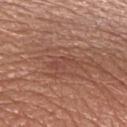Clinical impression:
Part of a total-body skin-imaging series; this lesion was reviewed on a skin check and was not flagged for biopsy.
Clinical summary:
On the left upper arm. Automated image analysis of the tile measured a footprint of about 4 mm², an outline eccentricity of about 0.95 (0 = round, 1 = elongated), and two-axis asymmetry of about 0.55. The software also gave a within-lesion color-variation index near 1/10 and radial color variation of about 0. A roughly 15 mm field-of-view crop from a total-body skin photograph. The recorded lesion diameter is about 4 mm. The patient is a male aged 53–57.